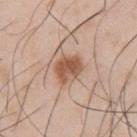Captured during whole-body skin photography for melanoma surveillance; the lesion was not biopsied. The lesion is located on the left upper arm. This image is a 15 mm lesion crop taken from a total-body photograph. A male patient, approximately 55 years of age.